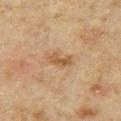biopsy status: catalogued during a skin exam; not biopsied
patient: male, aged 48–52
image-analysis metrics: a footprint of about 3.5 mm², an outline eccentricity of about 0.8 (0 = round, 1 = elongated), and a symmetry-axis asymmetry near 0.35
lesion size: about 3 mm
acquisition: ~15 mm crop, total-body skin-cancer survey
tile lighting: cross-polarized
body site: the arm